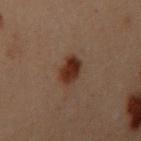Clinical impression: Part of a total-body skin-imaging series; this lesion was reviewed on a skin check and was not flagged for biopsy. Acquisition and patient details: The lesion is on the arm. A close-up tile cropped from a whole-body skin photograph, about 15 mm across. The subject is a female aged 28 to 32.